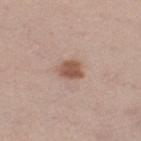Assessment:
Recorded during total-body skin imaging; not selected for excision or biopsy.
Image and clinical context:
The patient is a male about 60 years old. A 15 mm crop from a total-body photograph taken for skin-cancer surveillance. The lesion is located on the left thigh. The recorded lesion diameter is about 3 mm.